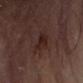Q: Is there a histopathology result?
A: catalogued during a skin exam; not biopsied
Q: Where on the body is the lesion?
A: the arm
Q: How was this image acquired?
A: ~15 mm tile from a whole-body skin photo
Q: Illumination type?
A: cross-polarized illumination
Q: What did automated image analysis measure?
A: a mean CIELAB color near L≈21 a*≈17 b*≈18, a lesion–skin lightness drop of about 6, and a normalized lesion–skin contrast near 8; a border-irregularity index near 3.5/10, internal color variation of about 2.5 on a 0–10 scale, and radial color variation of about 0.5
Q: What is the lesion's diameter?
A: ≈3.5 mm
Q: What are the patient's age and sex?
A: male, roughly 70 years of age